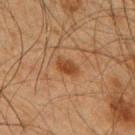Captured during whole-body skin photography for melanoma surveillance; the lesion was not biopsied. A 15 mm close-up tile from a total-body photography series done for melanoma screening. The lesion's longest dimension is about 3 mm. The patient is a male aged approximately 65. An algorithmic analysis of the crop reported a shape eccentricity near 0.85 and a shape-asymmetry score of about 0.25 (0 = symmetric). It also reported a lesion color around L≈37 a*≈20 b*≈31 in CIELAB, roughly 8 lightness units darker than nearby skin, and a normalized lesion–skin contrast near 7.5. It also reported a border-irregularity rating of about 2.5/10, a color-variation rating of about 2/10, and peripheral color asymmetry of about 0.5. The lesion is on the upper back. This is a cross-polarized tile.